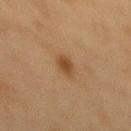No biopsy was performed on this lesion — it was imaged during a full skin examination and was not determined to be concerning. A female patient approximately 40 years of age. Located on the mid back. A region of skin cropped from a whole-body photographic capture, roughly 15 mm wide. The tile uses cross-polarized illumination. Approximately 2.5 mm at its widest. The lesion-visualizer software estimated an outline eccentricity of about 0.8 (0 = round, 1 = elongated) and a shape-asymmetry score of about 0.3 (0 = symmetric). The software also gave an average lesion color of about L≈39 a*≈17 b*≈32 (CIELAB) and a normalized border contrast of about 8. The analysis additionally found a within-lesion color-variation index near 1.5/10.